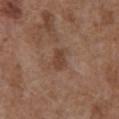The lesion was photographed on a routine skin check and not biopsied; there is no pathology result.
Located on the abdomen.
Imaged with white-light lighting.
A male patient, aged approximately 75.
A lesion tile, about 15 mm wide, cut from a 3D total-body photograph.
Automated tile analysis of the lesion measured a lesion area of about 4 mm² and a shape eccentricity near 0.8. The analysis additionally found an average lesion color of about L≈41 a*≈19 b*≈27 (CIELAB) and a lesion–skin lightness drop of about 8. It also reported a within-lesion color-variation index near 2/10.
Approximately 2.5 mm at its widest.